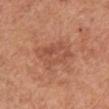The lesion was photographed on a routine skin check and not biopsied; there is no pathology result. The lesion is on the chest. A lesion tile, about 15 mm wide, cut from a 3D total-body photograph. Captured under white-light illumination. Measured at roughly 5.5 mm in maximum diameter. A male patient in their mid- to late 50s.